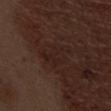Background:
A 15 mm close-up tile from a total-body photography series done for melanoma screening. A male subject, about 70 years old. The recorded lesion diameter is about 4 mm. The lesion is located on the abdomen. Automated tile analysis of the lesion measured a mean CIELAB color near L≈21 a*≈16 b*≈19. The analysis additionally found border irregularity of about 6 on a 0–10 scale and peripheral color asymmetry of about 0.5. It also reported a classifier nevus-likeness of about 0/100 and a detector confidence of about 100 out of 100 that the crop contains a lesion.